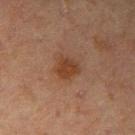Located on the right upper arm.
A region of skin cropped from a whole-body photographic capture, roughly 15 mm wide.
A male subject roughly 70 years of age.